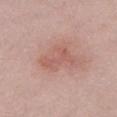Clinical impression:
Part of a total-body skin-imaging series; this lesion was reviewed on a skin check and was not flagged for biopsy.
Acquisition and patient details:
A close-up tile cropped from a whole-body skin photograph, about 15 mm across. A female patient, about 40 years old. This is a white-light tile. From the chest. Automated tile analysis of the lesion measured an average lesion color of about L≈57 a*≈24 b*≈25 (CIELAB) and a normalized border contrast of about 5.5.